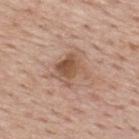lesion size = ≈4 mm
anatomic site = the upper back
patient = male, aged around 75
acquisition = 15 mm crop, total-body photography